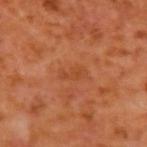Assessment:
This lesion was catalogued during total-body skin photography and was not selected for biopsy.
Clinical summary:
A male patient aged 58 to 62. The lesion is located on the left upper arm. A 15 mm crop from a total-body photograph taken for skin-cancer surveillance.